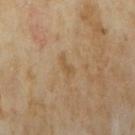This lesion was catalogued during total-body skin photography and was not selected for biopsy. The lesion is on the right upper arm. A close-up tile cropped from a whole-body skin photograph, about 15 mm across. A female subject in their mid- to late 30s.